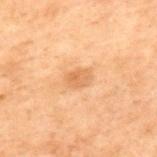No biopsy was performed on this lesion — it was imaged during a full skin examination and was not determined to be concerning.
The recorded lesion diameter is about 2.5 mm.
The tile uses cross-polarized illumination.
An algorithmic analysis of the crop reported a footprint of about 4.5 mm², a shape eccentricity near 0.75, and a shape-asymmetry score of about 0.25 (0 = symmetric). It also reported a mean CIELAB color near L≈51 a*≈19 b*≈34 and a lesion–skin lightness drop of about 7.
From the upper back.
The subject is a male aged approximately 70.
This image is a 15 mm lesion crop taken from a total-body photograph.